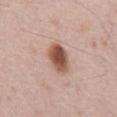• subject · male, approximately 65 years of age
• tile lighting · white-light illumination
• body site · the mid back
• automated metrics · a footprint of about 8 mm² and a symmetry-axis asymmetry near 0.15; an automated nevus-likeness rating near 100 out of 100 and lesion-presence confidence of about 100/100
• image · ~15 mm tile from a whole-body skin photo
• lesion size · about 4 mm